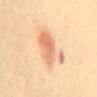Q: Is there a histopathology result?
A: catalogued during a skin exam; not biopsied
Q: What is the anatomic site?
A: the mid back
Q: How was this image acquired?
A: ~15 mm tile from a whole-body skin photo
Q: Who is the patient?
A: female, about 40 years old